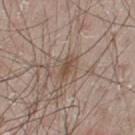Clinical summary:
A male subject aged approximately 65. The tile uses white-light illumination. The lesion is on the left upper arm. The lesion's longest dimension is about 3.5 mm. A close-up tile cropped from a whole-body skin photograph, about 15 mm across.